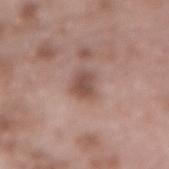Imaged during a routine full-body skin examination; the lesion was not biopsied and no histopathology is available.
A region of skin cropped from a whole-body photographic capture, roughly 15 mm wide.
This is a white-light tile.
The lesion-visualizer software estimated an area of roughly 5 mm² and a symmetry-axis asymmetry near 0.3. The software also gave a within-lesion color-variation index near 2/10.
The recorded lesion diameter is about 2.5 mm.
The subject is a male aged 53–57.
The lesion is on the lower back.